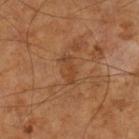Case summary:
- diameter — ~3.5 mm (longest diameter)
- body site — the left lower leg
- imaging modality — 15 mm crop, total-body photography
- image-analysis metrics — a lesion area of about 3.5 mm² and a shape eccentricity near 0.95; roughly 6 lightness units darker than nearby skin and a lesion-to-skin contrast of about 5.5 (normalized; higher = more distinct); a classifier nevus-likeness of about 0/100 and a lesion-detection confidence of about 100/100
- subject — male, aged approximately 65